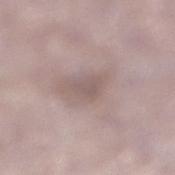notes = catalogued during a skin exam; not biopsied | anatomic site = the leg | automated lesion analysis = a shape eccentricity near 0.35 and a symmetry-axis asymmetry near 0.4; a nevus-likeness score of about 0/100 and a lesion-detection confidence of about 65/100 | image source = total-body-photography crop, ~15 mm field of view | lesion size = ~2.5 mm (longest diameter) | patient = male, in their mid- to late 70s | illumination = white-light.